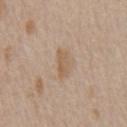<tbp_lesion>
  <biopsy_status>not biopsied; imaged during a skin examination</biopsy_status>
  <patient>
    <sex>male</sex>
    <age_approx>65</age_approx>
  </patient>
  <automated_metrics>
    <area_mm2_approx>4.0</area_mm2_approx>
    <eccentricity>0.9</eccentricity>
    <shape_asymmetry>0.3</shape_asymmetry>
    <cielab_L>58</cielab_L>
    <cielab_a>16</cielab_a>
    <cielab_b>32</cielab_b>
    <vs_skin_contrast_norm>6.5</vs_skin_contrast_norm>
    <nevus_likeness_0_100>0</nevus_likeness_0_100>
  </automated_metrics>
  <site>chest</site>
  <lesion_size>
    <long_diameter_mm_approx>3.5</long_diameter_mm_approx>
  </lesion_size>
  <image>
    <source>total-body photography crop</source>
    <field_of_view_mm>15</field_of_view_mm>
  </image>
</tbp_lesion>